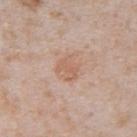Imaged during a routine full-body skin examination; the lesion was not biopsied and no histopathology is available. Captured under white-light illumination. A male subject, aged approximately 65. An algorithmic analysis of the crop reported an area of roughly 4.5 mm², an eccentricity of roughly 0.6, and a symmetry-axis asymmetry near 0.25. The analysis additionally found a lesion color around L≈60 a*≈20 b*≈30 in CIELAB and about 7 CIELAB-L* units darker than the surrounding skin. Measured at roughly 2.5 mm in maximum diameter. A lesion tile, about 15 mm wide, cut from a 3D total-body photograph. On the abdomen.